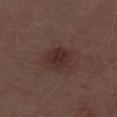This lesion was catalogued during total-body skin photography and was not selected for biopsy. The lesion is located on the left lower leg. A lesion tile, about 15 mm wide, cut from a 3D total-body photograph. A male subject, aged approximately 70. The total-body-photography lesion software estimated an area of roughly 6.5 mm², an outline eccentricity of about 0.5 (0 = round, 1 = elongated), and two-axis asymmetry of about 0.2. And it measured about 7 CIELAB-L* units darker than the surrounding skin and a normalized lesion–skin contrast near 7.5. The software also gave a lesion-detection confidence of about 100/100. This is a white-light tile. About 3 mm across.